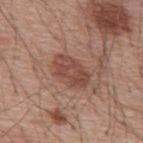notes = total-body-photography surveillance lesion; no biopsy | image = 15 mm crop, total-body photography | location = the mid back | TBP lesion metrics = an area of roughly 10 mm² and a symmetry-axis asymmetry near 0.2; a border-irregularity index near 2.5/10, internal color variation of about 3.5 on a 0–10 scale, and peripheral color asymmetry of about 1; an automated nevus-likeness rating near 55 out of 100 and a detector confidence of about 100 out of 100 that the crop contains a lesion | patient = male, aged around 55 | tile lighting = white-light illumination.